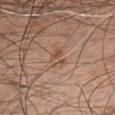This lesion was catalogued during total-body skin photography and was not selected for biopsy. This image is a 15 mm lesion crop taken from a total-body photograph. Captured under white-light illumination. On the chest. Automated image analysis of the tile measured an area of roughly 4 mm² and an outline eccentricity of about 0.6 (0 = round, 1 = elongated). It also reported a mean CIELAB color near L≈52 a*≈20 b*≈30 and a lesion–skin lightness drop of about 7. The analysis additionally found a border-irregularity rating of about 4/10 and a peripheral color-asymmetry measure near 2. A male subject, approximately 50 years of age. About 2.5 mm across.